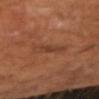workup: no biopsy performed (imaged during a skin exam)
lesion size: ≈3.5 mm
patient: male, approximately 60 years of age
tile lighting: cross-polarized illumination
location: the right forearm
acquisition: 15 mm crop, total-body photography
automated metrics: an area of roughly 6 mm², an eccentricity of roughly 0.85, and a shape-asymmetry score of about 0.25 (0 = symmetric)